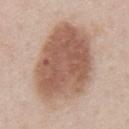Impression:
The lesion was photographed on a routine skin check and not biopsied; there is no pathology result.
Acquisition and patient details:
The lesion is on the front of the torso. A roughly 15 mm field-of-view crop from a total-body skin photograph. A male patient aged around 60.